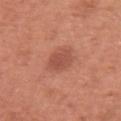biopsy status=no biopsy performed (imaged during a skin exam) | illumination=white-light illumination | imaging modality=~15 mm crop, total-body skin-cancer survey | subject=female, aged 48 to 52 | automated lesion analysis=an eccentricity of roughly 0.65 and two-axis asymmetry of about 0.2; a border-irregularity index near 2.5/10, a color-variation rating of about 1.5/10, and radial color variation of about 0.5 | size=~3 mm (longest diameter) | location=the right upper arm.